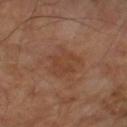Recorded during total-body skin imaging; not selected for excision or biopsy.
A male subject, in their mid- to late 60s.
A close-up tile cropped from a whole-body skin photograph, about 15 mm across.
The recorded lesion diameter is about 4.5 mm.
This is a cross-polarized tile.